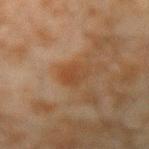<case>
  <site>arm</site>
  <image>
    <source>total-body photography crop</source>
    <field_of_view_mm>15</field_of_view_mm>
  </image>
  <patient>
    <sex>male</sex>
    <age_approx>45</age_approx>
  </patient>
</case>